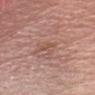Captured during whole-body skin photography for melanoma surveillance; the lesion was not biopsied.